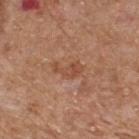The lesion was tiled from a total-body skin photograph and was not biopsied.
About 3.5 mm across.
Located on the upper back.
Captured under white-light illumination.
A 15 mm close-up tile from a total-body photography series done for melanoma screening.
A male subject roughly 65 years of age.